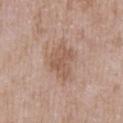Q: Was this lesion biopsied?
A: catalogued during a skin exam; not biopsied
Q: What lighting was used for the tile?
A: white-light
Q: Lesion location?
A: the chest
Q: What is the lesion's diameter?
A: ≈4.5 mm
Q: What are the patient's age and sex?
A: male, aged around 50
Q: Automated lesion metrics?
A: a lesion area of about 11 mm², a shape eccentricity near 0.7, and two-axis asymmetry of about 0.3; border irregularity of about 4 on a 0–10 scale, internal color variation of about 2.5 on a 0–10 scale, and radial color variation of about 1
Q: What kind of image is this?
A: total-body-photography crop, ~15 mm field of view